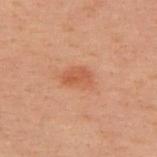Assessment: Part of a total-body skin-imaging series; this lesion was reviewed on a skin check and was not flagged for biopsy. Image and clinical context: Cropped from a total-body skin-imaging series; the visible field is about 15 mm. Imaged with cross-polarized lighting. Longest diameter approximately 3.5 mm. An algorithmic analysis of the crop reported a lesion color around L≈45 a*≈22 b*≈29 in CIELAB, about 7 CIELAB-L* units darker than the surrounding skin, and a normalized lesion–skin contrast near 5.5. And it measured a nevus-likeness score of about 80/100 and a lesion-detection confidence of about 100/100. The lesion is on the upper back. The patient is a male in their 40s.